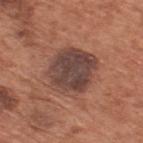The lesion was tiled from a total-body skin photograph and was not biopsied.
From the upper back.
The patient is a male in their mid- to late 60s.
A 15 mm close-up extracted from a 3D total-body photography capture.